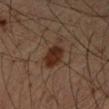{"lighting": "cross-polarized", "image": {"source": "total-body photography crop", "field_of_view_mm": 15}, "patient": {"sex": "male", "age_approx": 50}, "site": "right upper arm", "lesion_size": {"long_diameter_mm_approx": 4.0}, "automated_metrics": {"cielab_L": 25, "cielab_a": 16, "cielab_b": 23, "vs_skin_darker_L": 9.0, "vs_skin_contrast_norm": 10.0, "nevus_likeness_0_100": 90, "lesion_detection_confidence_0_100": 100}}